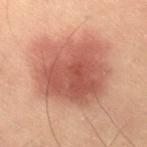Recorded during total-body skin imaging; not selected for excision or biopsy. A roughly 15 mm field-of-view crop from a total-body skin photograph. Longest diameter approximately 8 mm. Located on the back. A male subject, in their mid- to late 50s.